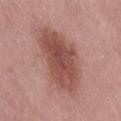biopsy status = imaged on a skin check; not biopsied | anatomic site = the right thigh | image = ~15 mm tile from a whole-body skin photo | lesion size = ~8.5 mm (longest diameter) | patient = female, aged 38 to 42 | automated metrics = border irregularity of about 2.5 on a 0–10 scale, a within-lesion color-variation index near 4/10, and a peripheral color-asymmetry measure near 1.